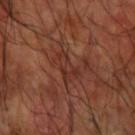Clinical impression:
No biopsy was performed on this lesion — it was imaged during a full skin examination and was not determined to be concerning.
Context:
The tile uses cross-polarized illumination. The lesion is located on the right forearm. Approximately 3.5 mm at its widest. A 15 mm close-up tile from a total-body photography series done for melanoma screening. A male patient about 60 years old.